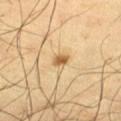Automated tile analysis of the lesion measured a lesion color around L≈62 a*≈18 b*≈43 in CIELAB, roughly 14 lightness units darker than nearby skin, and a normalized lesion–skin contrast near 9. The software also gave an automated nevus-likeness rating near 90 out of 100 and a lesion-detection confidence of about 100/100. Located on the right thigh. The subject is a male in their mid-60s. Cropped from a total-body skin-imaging series; the visible field is about 15 mm. The lesion's longest dimension is about 2 mm.